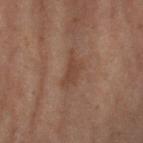Part of a total-body skin-imaging series; this lesion was reviewed on a skin check and was not flagged for biopsy.
Automated tile analysis of the lesion measured a border-irregularity rating of about 5.5/10, internal color variation of about 1 on a 0–10 scale, and radial color variation of about 0.5. The analysis additionally found a nevus-likeness score of about 0/100 and a lesion-detection confidence of about 95/100.
The patient is a male about 70 years old.
On the arm.
A 15 mm crop from a total-body photograph taken for skin-cancer surveillance.
Imaged with cross-polarized lighting.